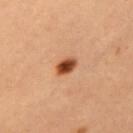workup: imaged on a skin check; not biopsied | illumination: cross-polarized | automated metrics: a lesion area of about 4 mm² and a shape-asymmetry score of about 0.15 (0 = symmetric); an average lesion color of about L≈38 a*≈22 b*≈31 (CIELAB), roughly 16 lightness units darker than nearby skin, and a normalized border contrast of about 12.5; an automated nevus-likeness rating near 100 out of 100 | subject: female, aged 38 to 42 | imaging modality: ~15 mm crop, total-body skin-cancer survey | location: the front of the torso | diameter: about 3 mm.